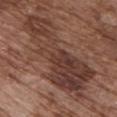{"biopsy_status": "not biopsied; imaged during a skin examination", "image": {"source": "total-body photography crop", "field_of_view_mm": 15}, "lighting": "white-light", "site": "front of the torso", "automated_metrics": {"area_mm2_approx": 40.0, "eccentricity": 0.95, "shape_asymmetry": 0.45, "border_irregularity_0_10": 7.5, "color_variation_0_10": 6.0, "peripheral_color_asymmetry": 2.0, "lesion_detection_confidence_0_100": 60}, "patient": {"sex": "male", "age_approx": 75}}